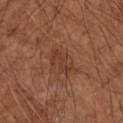Notes:
• notes · imaged on a skin check; not biopsied
• tile lighting · cross-polarized illumination
• image source · ~15 mm crop, total-body skin-cancer survey
• lesion diameter · ~3.5 mm (longest diameter)
• automated metrics · a lesion area of about 6.5 mm², an eccentricity of roughly 0.8, and a symmetry-axis asymmetry near 0.3; a border-irregularity rating of about 3.5/10, a color-variation rating of about 2.5/10, and peripheral color asymmetry of about 1
• patient · male, aged 63–67
• location · the right forearm